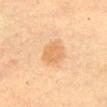Clinical impression:
No biopsy was performed on this lesion — it was imaged during a full skin examination and was not determined to be concerning.
Clinical summary:
The tile uses cross-polarized illumination. Cropped from a whole-body photographic skin survey; the tile spans about 15 mm. A female patient, about 60 years old. An algorithmic analysis of the crop reported a border-irregularity rating of about 2/10 and peripheral color asymmetry of about 0.5. Located on the chest. Approximately 4 mm at its widest.